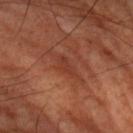Clinical summary:
Captured under cross-polarized illumination. A male subject, approximately 65 years of age. A close-up tile cropped from a whole-body skin photograph, about 15 mm across. From the left upper arm.